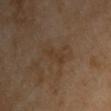Assessment: No biopsy was performed on this lesion — it was imaged during a full skin examination and was not determined to be concerning. Acquisition and patient details: A male patient aged approximately 55. Longest diameter approximately 3 mm. An algorithmic analysis of the crop reported a lesion area of about 3 mm², a shape eccentricity near 0.95, and a symmetry-axis asymmetry near 0.35. The lesion is located on the chest. The tile uses cross-polarized illumination. A lesion tile, about 15 mm wide, cut from a 3D total-body photograph.